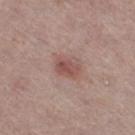Impression: Imaged during a routine full-body skin examination; the lesion was not biopsied and no histopathology is available. Acquisition and patient details: Located on the right thigh. A close-up tile cropped from a whole-body skin photograph, about 15 mm across. A female patient aged around 60.